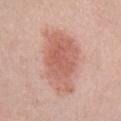Impression: No biopsy was performed on this lesion — it was imaged during a full skin examination and was not determined to be concerning. Image and clinical context: Captured under white-light illumination. From the chest. The lesion's longest dimension is about 8 mm. A close-up tile cropped from a whole-body skin photograph, about 15 mm across. The subject is a female in their mid-40s.